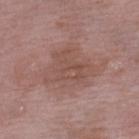The lesion was photographed on a routine skin check and not biopsied; there is no pathology result. Automated tile analysis of the lesion measured a lesion area of about 17 mm², a shape eccentricity near 0.55, and a shape-asymmetry score of about 0.4 (0 = symmetric). It also reported an average lesion color of about L≈50 a*≈20 b*≈24 (CIELAB), roughly 7 lightness units darker than nearby skin, and a normalized lesion–skin contrast near 5.5. And it measured an automated nevus-likeness rating near 0 out of 100 and a lesion-detection confidence of about 100/100. Captured under white-light illumination. The patient is a female approximately 70 years of age. A 15 mm close-up extracted from a 3D total-body photography capture. Located on the left lower leg. The lesion's longest dimension is about 5.5 mm.